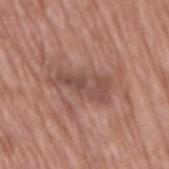Recorded during total-body skin imaging; not selected for excision or biopsy.
Captured under white-light illumination.
An algorithmic analysis of the crop reported a lesion color around L≈49 a*≈20 b*≈24 in CIELAB and roughly 9 lightness units darker than nearby skin. It also reported border irregularity of about 6.5 on a 0–10 scale and a peripheral color-asymmetry measure near 1.5. The analysis additionally found a classifier nevus-likeness of about 0/100 and a lesion-detection confidence of about 90/100.
The lesion is on the mid back.
About 6 mm across.
A female subject aged 73–77.
Cropped from a total-body skin-imaging series; the visible field is about 15 mm.